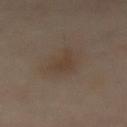| field | value |
|---|---|
| biopsy status | no biopsy performed (imaged during a skin exam) |
| image | 15 mm crop, total-body photography |
| location | the abdomen |
| lighting | cross-polarized |
| automated metrics | a lesion color around L≈39 a*≈13 b*≈25 in CIELAB, roughly 5 lightness units darker than nearby skin, and a normalized lesion–skin contrast near 5.5 |
| size | ~3.5 mm (longest diameter) |